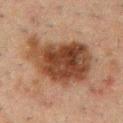notes: total-body-photography surveillance lesion; no biopsy | image-analysis metrics: a shape-asymmetry score of about 0.3 (0 = symmetric); a mean CIELAB color near L≈34 a*≈17 b*≈26 and a lesion–skin lightness drop of about 12; a border-irregularity index near 4/10, a within-lesion color-variation index near 5.5/10, and peripheral color asymmetry of about 1.5 | body site: the chest | acquisition: 15 mm crop, total-body photography | patient: male, aged 48 to 52 | illumination: cross-polarized | lesion diameter: ≈8 mm.